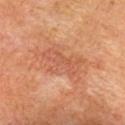Q: Was this lesion biopsied?
A: no biopsy performed (imaged during a skin exam)
Q: What lighting was used for the tile?
A: cross-polarized illumination
Q: What kind of image is this?
A: 15 mm crop, total-body photography
Q: Patient demographics?
A: female, in their mid- to late 50s
Q: What is the anatomic site?
A: the head or neck